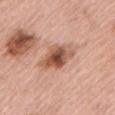<tbp_lesion>
  <biopsy_status>not biopsied; imaged during a skin examination</biopsy_status>
  <lesion_size>
    <long_diameter_mm_approx>4.5</long_diameter_mm_approx>
  </lesion_size>
  <lighting>white-light</lighting>
  <site>left upper arm</site>
  <patient>
    <sex>male</sex>
    <age_approx>75</age_approx>
  </patient>
  <image>
    <source>total-body photography crop</source>
    <field_of_view_mm>15</field_of_view_mm>
  </image>
</tbp_lesion>